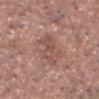<record>
  <biopsy_status>not biopsied; imaged during a skin examination</biopsy_status>
  <site>head or neck</site>
  <patient>
    <sex>male</sex>
    <age_approx>60</age_approx>
  </patient>
  <lesion_size>
    <long_diameter_mm_approx>5.0</long_diameter_mm_approx>
  </lesion_size>
  <lighting>white-light</lighting>
  <image>
    <source>total-body photography crop</source>
    <field_of_view_mm>15</field_of_view_mm>
  </image>
</record>